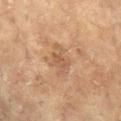{
  "biopsy_status": "not biopsied; imaged during a skin examination",
  "site": "arm",
  "image": {
    "source": "total-body photography crop",
    "field_of_view_mm": 15
  },
  "lighting": "cross-polarized",
  "patient": {
    "sex": "female",
    "age_approx": 80
  }
}